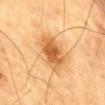workup=catalogued during a skin exam; not biopsied | diameter=≈5 mm | location=the chest | patient=male, aged approximately 85 or older | lighting=cross-polarized illumination | acquisition=total-body-photography crop, ~15 mm field of view.